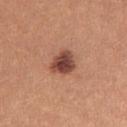notes — imaged on a skin check; not biopsied.